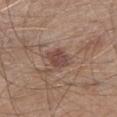- biopsy status — total-body-photography surveillance lesion; no biopsy